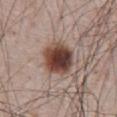Impression: Captured during whole-body skin photography for melanoma surveillance; the lesion was not biopsied. Background: The total-body-photography lesion software estimated a lesion area of about 14 mm², an eccentricity of roughly 0.3, and a symmetry-axis asymmetry near 0.15. It also reported border irregularity of about 1.5 on a 0–10 scale, a within-lesion color-variation index near 8/10, and peripheral color asymmetry of about 2. A roughly 15 mm field-of-view crop from a total-body skin photograph. Approximately 4.5 mm at its widest. This is a white-light tile. On the abdomen. A male subject, aged 63 to 67.